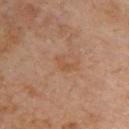biopsy status = catalogued during a skin exam; not biopsied | tile lighting = cross-polarized | site = the upper back | image source = ~15 mm tile from a whole-body skin photo | patient = female, approximately 60 years of age.